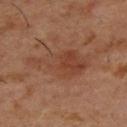Q: How was this image acquired?
A: ~15 mm tile from a whole-body skin photo
Q: Illumination type?
A: cross-polarized
Q: What is the anatomic site?
A: the upper back
Q: How large is the lesion?
A: about 7.5 mm
Q: Who is the patient?
A: male, roughly 55 years of age